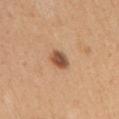Clinical impression: This lesion was catalogued during total-body skin photography and was not selected for biopsy. Context: A female patient, aged approximately 45. The lesion is on the arm. Cropped from a total-body skin-imaging series; the visible field is about 15 mm. Captured under white-light illumination. The lesion's longest dimension is about 2.5 mm.